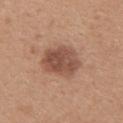No biopsy was performed on this lesion — it was imaged during a full skin examination and was not determined to be concerning.
Imaged with white-light lighting.
The lesion is on the upper back.
Automated tile analysis of the lesion measured a border-irregularity index near 2/10 and internal color variation of about 4 on a 0–10 scale.
A female subject, in their mid- to late 40s.
A roughly 15 mm field-of-view crop from a total-body skin photograph.
Measured at roughly 5 mm in maximum diameter.